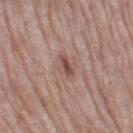Assessment: No biopsy was performed on this lesion — it was imaged during a full skin examination and was not determined to be concerning. Image and clinical context: A female patient, approximately 75 years of age. About 3 mm across. Imaged with white-light lighting. Located on the left thigh. Cropped from a whole-body photographic skin survey; the tile spans about 15 mm.